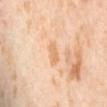Assessment:
This lesion was catalogued during total-body skin photography and was not selected for biopsy.
Image and clinical context:
A female subject, approximately 55 years of age. From the lower back. A close-up tile cropped from a whole-body skin photograph, about 15 mm across.